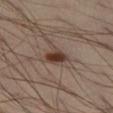biopsy_status: not biopsied; imaged during a skin examination
lesion_size:
  long_diameter_mm_approx: 2.5
patient:
  sex: male
  age_approx: 40
automated_metrics:
  eccentricity: 0.45
  shape_asymmetry: 0.2
  cielab_L: 36
  cielab_a: 17
  cielab_b: 24
  vs_skin_darker_L: 12.0
  vs_skin_contrast_norm: 10.5
  border_irregularity_0_10: 2.0
  color_variation_0_10: 4.5
  peripheral_color_asymmetry: 1.5
  nevus_likeness_0_100: 100
  lesion_detection_confidence_0_100: 100
image:
  source: total-body photography crop
  field_of_view_mm: 15
lighting: cross-polarized
site: left thigh